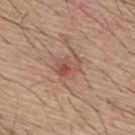The lesion was tiled from a total-body skin photograph and was not biopsied.
About 3.5 mm across.
A male subject in their mid- to late 60s.
The lesion is on the upper back.
Cropped from a whole-body photographic skin survey; the tile spans about 15 mm.